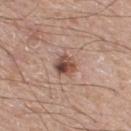{"biopsy_status": "not biopsied; imaged during a skin examination", "site": "right thigh", "image": {"source": "total-body photography crop", "field_of_view_mm": 15}, "patient": {"sex": "male", "age_approx": 60}}